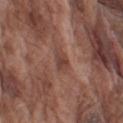Recorded during total-body skin imaging; not selected for excision or biopsy. A 15 mm close-up tile from a total-body photography series done for melanoma screening. A male subject about 75 years old. Approximately 3 mm at its widest. The tile uses white-light illumination. The lesion is on the left upper arm.